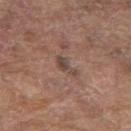Q: Was this lesion biopsied?
A: imaged on a skin check; not biopsied
Q: Illumination type?
A: white-light
Q: What are the patient's age and sex?
A: male, aged approximately 80
Q: What did automated image analysis measure?
A: a border-irregularity index near 4.5/10, internal color variation of about 1.5 on a 0–10 scale, and radial color variation of about 0.5
Q: What is the imaging modality?
A: ~15 mm crop, total-body skin-cancer survey
Q: Where on the body is the lesion?
A: the upper back
Q: Lesion size?
A: ≈3 mm